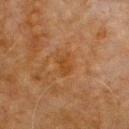This lesion was catalogued during total-body skin photography and was not selected for biopsy.
This is a cross-polarized tile.
The lesion is located on the front of the torso.
A male patient in their 80s.
An algorithmic analysis of the crop reported an average lesion color of about L≈35 a*≈20 b*≈33 (CIELAB) and a lesion-to-skin contrast of about 6 (normalized; higher = more distinct).
A roughly 15 mm field-of-view crop from a total-body skin photograph.